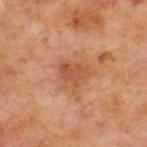{
  "biopsy_status": "not biopsied; imaged during a skin examination",
  "lighting": "cross-polarized",
  "patient": {
    "sex": "male",
    "age_approx": 65
  },
  "image": {
    "source": "total-body photography crop",
    "field_of_view_mm": 15
  },
  "automated_metrics": {
    "area_mm2_approx": 7.0,
    "shape_asymmetry": 0.3,
    "cielab_L": 41,
    "cielab_a": 21,
    "cielab_b": 30,
    "vs_skin_darker_L": 7.0,
    "vs_skin_contrast_norm": 6.0,
    "lesion_detection_confidence_0_100": 100
  },
  "site": "chest"
}